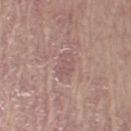| feature | finding |
|---|---|
| image source | 15 mm crop, total-body photography |
| site | the left upper arm |
| subject | male, in their mid- to late 60s |